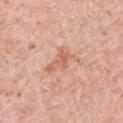<tbp_lesion>
  <biopsy_status>not biopsied; imaged during a skin examination</biopsy_status>
  <lesion_size>
    <long_diameter_mm_approx>4.5</long_diameter_mm_approx>
  </lesion_size>
  <image>
    <source>total-body photography crop</source>
    <field_of_view_mm>15</field_of_view_mm>
  </image>
  <patient>
    <sex>male</sex>
    <age_approx>80</age_approx>
  </patient>
  <site>left upper arm</site>
  <automated_metrics>
    <area_mm2_approx>7.0</area_mm2_approx>
    <eccentricity>0.85</eccentricity>
    <shape_asymmetry>0.5</shape_asymmetry>
    <border_irregularity_0_10>6.5</border_irregularity_0_10>
    <color_variation_0_10>2.5</color_variation_0_10>
    <peripheral_color_asymmetry>1.0</peripheral_color_asymmetry>
    <nevus_likeness_0_100>0</nevus_likeness_0_100>
    <lesion_detection_confidence_0_100>100</lesion_detection_confidence_0_100>
  </automated_metrics>
  <lighting>white-light</lighting>
</tbp_lesion>